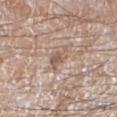notes = catalogued during a skin exam; not biopsied | diameter = ≈3 mm | anatomic site = the left lower leg | subject = male, aged 58 to 62 | image-analysis metrics = a shape eccentricity near 0.75 and a symmetry-axis asymmetry near 0.4; a lesion-detection confidence of about 65/100 | imaging modality = total-body-photography crop, ~15 mm field of view.